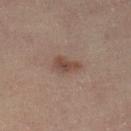Q: What are the patient's age and sex?
A: male, approximately 55 years of age
Q: How was this image acquired?
A: total-body-photography crop, ~15 mm field of view
Q: What did automated image analysis measure?
A: an outline eccentricity of about 0.85 (0 = round, 1 = elongated) and a symmetry-axis asymmetry near 0.3; an automated nevus-likeness rating near 40 out of 100 and lesion-presence confidence of about 100/100
Q: What is the anatomic site?
A: the left lower leg
Q: Illumination type?
A: cross-polarized